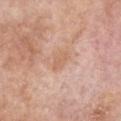Notes:
– follow-up · imaged on a skin check; not biopsied
– site · the chest
– size · ≈3 mm
– imaging modality · total-body-photography crop, ~15 mm field of view
– patient · female, in their mid-70s